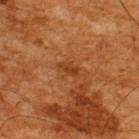Automated image analysis of the tile measured a lesion color around L≈33 a*≈23 b*≈34 in CIELAB, about 6 CIELAB-L* units darker than the surrounding skin, and a normalized lesion–skin contrast near 6. The lesion is located on the upper back. A male patient aged around 65. A lesion tile, about 15 mm wide, cut from a 3D total-body photograph.